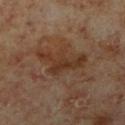  biopsy_status: not biopsied; imaged during a skin examination
  lighting: cross-polarized
  site: leg
  patient:
    sex: male
    age_approx: 60
  lesion_size:
    long_diameter_mm_approx: 6.0
  automated_metrics:
    eccentricity: 0.9
    shape_asymmetry: 0.6
    cielab_L: 31
    cielab_a: 17
    cielab_b: 27
    vs_skin_darker_L: 7.0
    vs_skin_contrast_norm: 8.0
    border_irregularity_0_10: 7.5
    peripheral_color_asymmetry: 0.5
  image:
    source: total-body photography crop
    field_of_view_mm: 15